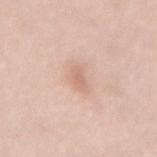The lesion was photographed on a routine skin check and not biopsied; there is no pathology result. The subject is a female roughly 65 years of age. Imaged with white-light lighting. About 2.5 mm across. A roughly 15 mm field-of-view crop from a total-body skin photograph. The lesion is located on the back.